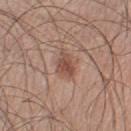Assessment:
Imaged during a routine full-body skin examination; the lesion was not biopsied and no histopathology is available.
Acquisition and patient details:
Cropped from a total-body skin-imaging series; the visible field is about 15 mm. Imaged with white-light lighting. From the left lower leg. Automated image analysis of the tile measured a border-irregularity rating of about 2.5/10, internal color variation of about 3.5 on a 0–10 scale, and peripheral color asymmetry of about 1. Longest diameter approximately 3.5 mm. A male subject, approximately 30 years of age.